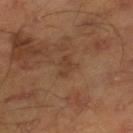biopsy status: total-body-photography surveillance lesion; no biopsy
lesion diameter: ~3 mm (longest diameter)
location: the right lower leg
automated lesion analysis: an average lesion color of about L≈39 a*≈19 b*≈30 (CIELAB), about 6 CIELAB-L* units darker than the surrounding skin, and a lesion-to-skin contrast of about 5 (normalized; higher = more distinct); a border-irregularity index near 3.5/10 and peripheral color asymmetry of about 0.5
patient: male, approximately 45 years of age
illumination: cross-polarized
acquisition: ~15 mm tile from a whole-body skin photo